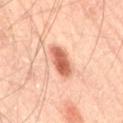- workup: total-body-photography surveillance lesion; no biopsy
- site: the right thigh
- lesion size: ~4 mm (longest diameter)
- imaging modality: 15 mm crop, total-body photography
- subject: male, aged 63–67